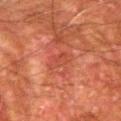Findings:
- biopsy status — imaged on a skin check; not biopsied
- subject — male, aged 78 to 82
- body site — the right upper arm
- imaging modality — ~15 mm tile from a whole-body skin photo
- tile lighting — cross-polarized illumination
- diameter — ~3 mm (longest diameter)
- image-analysis metrics — an area of roughly 4.5 mm², an outline eccentricity of about 0.7 (0 = round, 1 = elongated), and a shape-asymmetry score of about 0.3 (0 = symmetric); an average lesion color of about L≈38 a*≈28 b*≈29 (CIELAB), about 5 CIELAB-L* units darker than the surrounding skin, and a normalized border contrast of about 4.5; a border-irregularity rating of about 3/10, a color-variation rating of about 2.5/10, and a peripheral color-asymmetry measure near 1; an automated nevus-likeness rating near 0 out of 100 and lesion-presence confidence of about 95/100